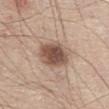biopsy status = catalogued during a skin exam; not biopsied
size = about 5 mm
subject = male, roughly 80 years of age
body site = the abdomen
imaging modality = total-body-photography crop, ~15 mm field of view